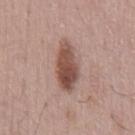  biopsy_status: not biopsied; imaged during a skin examination